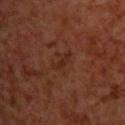notes=total-body-photography surveillance lesion; no biopsy | subject=male, aged 68–72 | anatomic site=the upper back | image=~15 mm crop, total-body skin-cancer survey.